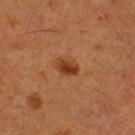Imaged during a routine full-body skin examination; the lesion was not biopsied and no histopathology is available.
Captured under cross-polarized illumination.
The patient is a male aged approximately 50.
Measured at roughly 2.5 mm in maximum diameter.
A lesion tile, about 15 mm wide, cut from a 3D total-body photograph.
The lesion is on the right thigh.